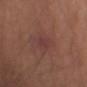<tbp_lesion>
  <biopsy_status>not biopsied; imaged during a skin examination</biopsy_status>
  <patient>
    <sex>male</sex>
    <age_approx>80</age_approx>
  </patient>
  <lighting>white-light</lighting>
  <image>
    <source>total-body photography crop</source>
    <field_of_view_mm>15</field_of_view_mm>
  </image>
  <site>left arm</site>
</tbp_lesion>